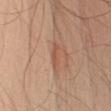• workup · total-body-photography surveillance lesion; no biopsy
• automated lesion analysis · an area of roughly 3.5 mm², an eccentricity of roughly 0.95, and a shape-asymmetry score of about 0.3 (0 = symmetric)
• subject · male, in their mid- to late 30s
• site · the left upper arm
• illumination · white-light
• size · ~3.5 mm (longest diameter)
• acquisition · ~15 mm tile from a whole-body skin photo